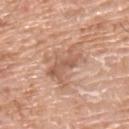Findings:
* notes: no biopsy performed (imaged during a skin exam)
* patient: male, in their mid-70s
* imaging modality: total-body-photography crop, ~15 mm field of view
* automated lesion analysis: an eccentricity of roughly 0.9 and two-axis asymmetry of about 0.4; a lesion color around L≈58 a*≈22 b*≈30 in CIELAB, roughly 10 lightness units darker than nearby skin, and a normalized lesion–skin contrast near 6.5; an automated nevus-likeness rating near 0 out of 100
* size: about 5 mm
* site: the upper back